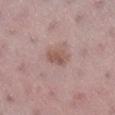{
  "biopsy_status": "not biopsied; imaged during a skin examination",
  "image": {
    "source": "total-body photography crop",
    "field_of_view_mm": 15
  },
  "lesion_size": {
    "long_diameter_mm_approx": 3.0
  },
  "site": "right lower leg",
  "lighting": "white-light",
  "patient": {
    "sex": "female",
    "age_approx": 45
  }
}